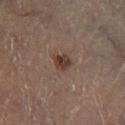Recorded during total-body skin imaging; not selected for excision or biopsy. Cropped from a whole-body photographic skin survey; the tile spans about 15 mm. A male patient approximately 60 years of age. Located on the left lower leg.